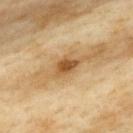Findings:
- tile lighting — cross-polarized illumination
- diameter — about 3 mm
- patient — female, aged 58–62
- image source — ~15 mm tile from a whole-body skin photo
- location — the upper back